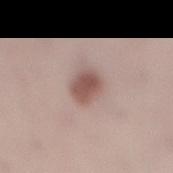Imaged during a routine full-body skin examination; the lesion was not biopsied and no histopathology is available.
A female subject in their mid-20s.
Imaged with white-light lighting.
Approximately 3.5 mm at its widest.
A lesion tile, about 15 mm wide, cut from a 3D total-body photograph.
On the right lower leg.